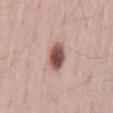notes: total-body-photography surveillance lesion; no biopsy | image: ~15 mm crop, total-body skin-cancer survey | automated lesion analysis: a lesion area of about 7 mm², an outline eccentricity of about 0.7 (0 = round, 1 = elongated), and a shape-asymmetry score of about 0.15 (0 = symmetric); a mean CIELAB color near L≈51 a*≈21 b*≈24, a lesion–skin lightness drop of about 19, and a normalized lesion–skin contrast near 12; a nevus-likeness score of about 100/100 | tile lighting: white-light | location: the lower back | patient: male, about 35 years old | lesion size: ≈3.5 mm.